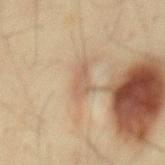Q: Was a biopsy performed?
A: catalogued during a skin exam; not biopsied
Q: Lesion size?
A: ≈3.5 mm
Q: What are the patient's age and sex?
A: male, in their 50s
Q: How was this image acquired?
A: 15 mm crop, total-body photography
Q: Where on the body is the lesion?
A: the mid back
Q: What lighting was used for the tile?
A: cross-polarized illumination